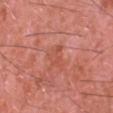workup — imaged on a skin check; not biopsied | lesion diameter — ≈3 mm | tile lighting — white-light | imaging modality — 15 mm crop, total-body photography | site — the head or neck | automated lesion analysis — an average lesion color of about L≈53 a*≈30 b*≈33 (CIELAB), roughly 5 lightness units darker than nearby skin, and a lesion-to-skin contrast of about 4.5 (normalized; higher = more distinct); a border-irregularity index near 4.5/10 and peripheral color asymmetry of about 0.5; an automated nevus-likeness rating near 0 out of 100 | subject — male, about 45 years old.